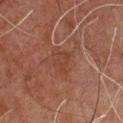Clinical impression:
Imaged during a routine full-body skin examination; the lesion was not biopsied and no histopathology is available.
Context:
From the chest. A lesion tile, about 15 mm wide, cut from a 3D total-body photograph. An algorithmic analysis of the crop reported an area of roughly 5 mm², a shape eccentricity near 0.65, and two-axis asymmetry of about 0.4. And it measured a mean CIELAB color near L≈30 a*≈18 b*≈23, a lesion–skin lightness drop of about 4, and a normalized border contrast of about 4.5. The analysis additionally found a nevus-likeness score of about 0/100. A male subject aged 58–62. This is a cross-polarized tile.